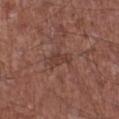This lesion was catalogued during total-body skin photography and was not selected for biopsy. The lesion is located on the right lower leg. Cropped from a total-body skin-imaging series; the visible field is about 15 mm. Captured under white-light illumination. A male patient aged 63 to 67.